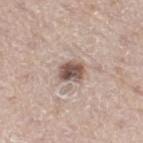workup: no biopsy performed (imaged during a skin exam) | image source: ~15 mm tile from a whole-body skin photo | tile lighting: white-light illumination | body site: the right thigh | patient: male, aged around 65.